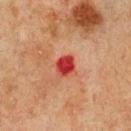– notes · total-body-photography surveillance lesion; no biopsy
– patient · male, aged 63–67
– diameter · ~2.5 mm (longest diameter)
– tile lighting · cross-polarized
– imaging modality · total-body-photography crop, ~15 mm field of view
– body site · the chest
– automated metrics · a footprint of about 4.5 mm² and two-axis asymmetry of about 0.2; about 14 CIELAB-L* units darker than the surrounding skin and a normalized lesion–skin contrast near 11.5; a border-irregularity rating of about 1.5/10, a within-lesion color-variation index near 2.5/10, and radial color variation of about 1; lesion-presence confidence of about 100/100